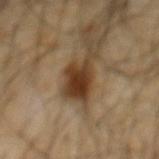Imaged during a routine full-body skin examination; the lesion was not biopsied and no histopathology is available.
Captured under cross-polarized illumination.
A male patient, approximately 65 years of age.
Measured at roughly 5.5 mm in maximum diameter.
The lesion-visualizer software estimated a lesion area of about 10 mm², an outline eccentricity of about 0.85 (0 = round, 1 = elongated), and a shape-asymmetry score of about 0.3 (0 = symmetric). And it measured a mean CIELAB color near L≈34 a*≈16 b*≈29, about 14 CIELAB-L* units darker than the surrounding skin, and a normalized lesion–skin contrast near 12.5. And it measured a detector confidence of about 100 out of 100 that the crop contains a lesion.
The lesion is on the mid back.
A region of skin cropped from a whole-body photographic capture, roughly 15 mm wide.